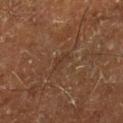Part of a total-body skin-imaging series; this lesion was reviewed on a skin check and was not flagged for biopsy. On the right lower leg. A male subject, roughly 65 years of age. The tile uses cross-polarized illumination. This image is a 15 mm lesion crop taken from a total-body photograph. Approximately 2.5 mm at its widest.